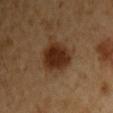notes: imaged on a skin check; not biopsied.